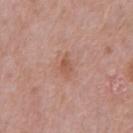Assessment:
No biopsy was performed on this lesion — it was imaged during a full skin examination and was not determined to be concerning.
Background:
The tile uses white-light illumination. A lesion tile, about 15 mm wide, cut from a 3D total-body photograph. The subject is a male aged around 70. The total-body-photography lesion software estimated a footprint of about 3.5 mm², an eccentricity of roughly 0.8, and two-axis asymmetry of about 0.35. The software also gave border irregularity of about 3 on a 0–10 scale, a within-lesion color-variation index near 2/10, and radial color variation of about 1. And it measured a nevus-likeness score of about 0/100 and lesion-presence confidence of about 100/100. The lesion is on the front of the torso. Approximately 2.5 mm at its widest.